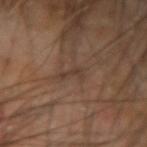biopsy_status: not biopsied; imaged during a skin examination
lighting: cross-polarized
patient:
  sex: male
  age_approx: 65
site: left forearm
image:
  source: total-body photography crop
  field_of_view_mm: 15
lesion_size:
  long_diameter_mm_approx: 2.5